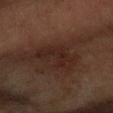<lesion>
  <biopsy_status>not biopsied; imaged during a skin examination</biopsy_status>
  <image>
    <source>total-body photography crop</source>
    <field_of_view_mm>15</field_of_view_mm>
  </image>
  <lighting>cross-polarized</lighting>
  <patient>
    <sex>male</sex>
    <age_approx>60</age_approx>
  </patient>
  <lesion_size>
    <long_diameter_mm_approx>5.5</long_diameter_mm_approx>
  </lesion_size>
  <site>right forearm</site>
  <automated_metrics>
    <nevus_likeness_0_100>0</nevus_likeness_0_100>
    <lesion_detection_confidence_0_100>100</lesion_detection_confidence_0_100>
  </automated_metrics>
</lesion>